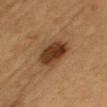Impression: This lesion was catalogued during total-body skin photography and was not selected for biopsy. Clinical summary: The lesion is on the arm. The subject is a male in their mid-80s or older. This image is a 15 mm lesion crop taken from a total-body photograph.